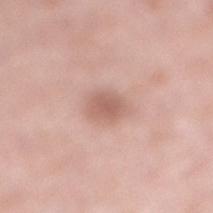biopsy_status: not biopsied; imaged during a skin examination
lesion_size:
  long_diameter_mm_approx: 3.0
site: right lower leg
image:
  source: total-body photography crop
  field_of_view_mm: 15
patient:
  sex: male
  age_approx: 50
lighting: white-light